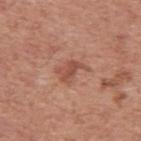Recorded during total-body skin imaging; not selected for excision or biopsy. From the upper back. A close-up tile cropped from a whole-body skin photograph, about 15 mm across. Captured under white-light illumination. Measured at roughly 3.5 mm in maximum diameter. A male subject aged approximately 55.